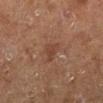Recorded during total-body skin imaging; not selected for excision or biopsy.